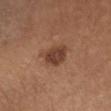Q: Was this lesion biopsied?
A: no biopsy performed (imaged during a skin exam)
Q: Where on the body is the lesion?
A: the chest
Q: Automated lesion metrics?
A: an area of roughly 8 mm², an outline eccentricity of about 0.5 (0 = round, 1 = elongated), and a shape-asymmetry score of about 0.2 (0 = symmetric); a lesion color around L≈42 a*≈21 b*≈29 in CIELAB, roughly 11 lightness units darker than nearby skin, and a lesion-to-skin contrast of about 8.5 (normalized; higher = more distinct); a nevus-likeness score of about 75/100 and a detector confidence of about 100 out of 100 that the crop contains a lesion
Q: Patient demographics?
A: female, aged 58 to 62
Q: What is the lesion's diameter?
A: ≈3.5 mm
Q: Illumination type?
A: white-light
Q: What is the imaging modality?
A: ~15 mm crop, total-body skin-cancer survey